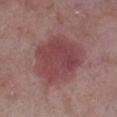Impression:
Captured during whole-body skin photography for melanoma surveillance; the lesion was not biopsied.
Acquisition and patient details:
A male patient aged around 75. A region of skin cropped from a whole-body photographic capture, roughly 15 mm wide. Imaged with white-light lighting. About 6.5 mm across. From the right lower leg.